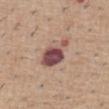Acquisition and patient details: The lesion is located on the abdomen. A male patient aged 58–62. A lesion tile, about 15 mm wide, cut from a 3D total-body photograph. Measured at roughly 4 mm in maximum diameter. Imaged with white-light lighting.